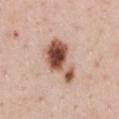Assessment:
Part of a total-body skin-imaging series; this lesion was reviewed on a skin check and was not flagged for biopsy.
Context:
The subject is a female aged around 45. The lesion-visualizer software estimated border irregularity of about 4.5 on a 0–10 scale. Longest diameter approximately 6 mm. This is a white-light tile. Cropped from a total-body skin-imaging series; the visible field is about 15 mm. From the chest.